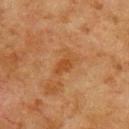This lesion was catalogued during total-body skin photography and was not selected for biopsy. The subject is a male aged around 80. The total-body-photography lesion software estimated a lesion area of about 4.5 mm². And it measured an automated nevus-likeness rating near 0 out of 100 and lesion-presence confidence of about 100/100. The lesion's longest dimension is about 3 mm. A roughly 15 mm field-of-view crop from a total-body skin photograph. The lesion is located on the upper back. Captured under cross-polarized illumination.